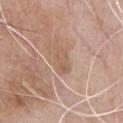{"biopsy_status": "not biopsied; imaged during a skin examination", "site": "chest", "lighting": "white-light", "image": {"source": "total-body photography crop", "field_of_view_mm": 15}, "automated_metrics": {"color_variation_0_10": 2.5, "peripheral_color_asymmetry": 1.0}, "patient": {"sex": "male", "age_approx": 70}, "lesion_size": {"long_diameter_mm_approx": 3.5}}